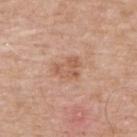No biopsy was performed on this lesion — it was imaged during a full skin examination and was not determined to be concerning. The recorded lesion diameter is about 3.5 mm. Located on the upper back. A roughly 15 mm field-of-view crop from a total-body skin photograph. Imaged with white-light lighting. A male patient, roughly 65 years of age.